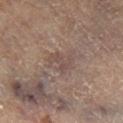Impression: Recorded during total-body skin imaging; not selected for excision or biopsy. Context: Imaged with cross-polarized lighting. The subject is a female in their 60s. A 15 mm crop from a total-body photograph taken for skin-cancer surveillance. From the right lower leg. The lesion-visualizer software estimated a symmetry-axis asymmetry near 0.4. The software also gave an average lesion color of about L≈47 a*≈16 b*≈21 (CIELAB), roughly 6 lightness units darker than nearby skin, and a normalized border contrast of about 5. The analysis additionally found a border-irregularity index near 4/10, a within-lesion color-variation index near 2/10, and radial color variation of about 0.5. It also reported a classifier nevus-likeness of about 0/100. Longest diameter approximately 3 mm.